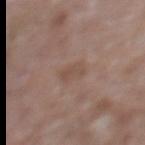The lesion was photographed on a routine skin check and not biopsied; there is no pathology result.
Measured at roughly 3 mm in maximum diameter.
The lesion is on the right lower leg.
Imaged with white-light lighting.
A male subject aged around 60.
A region of skin cropped from a whole-body photographic capture, roughly 15 mm wide.